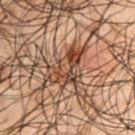| feature | finding |
|---|---|
| notes | no biopsy performed (imaged during a skin exam) |
| location | the arm |
| lighting | cross-polarized |
| imaging modality | total-body-photography crop, ~15 mm field of view |
| lesion diameter | about 6 mm |
| subject | male, approximately 50 years of age |